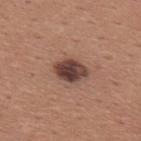body site — the upper back
size — about 4 mm
automated lesion analysis — a footprint of about 7.5 mm², a shape eccentricity near 0.75, and a symmetry-axis asymmetry near 0.2; a lesion-to-skin contrast of about 12.5 (normalized; higher = more distinct)
image — total-body-photography crop, ~15 mm field of view
lighting — white-light illumination
subject — male, aged approximately 45